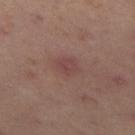Recorded during total-body skin imaging; not selected for excision or biopsy. The tile uses cross-polarized illumination. The lesion-visualizer software estimated an outline eccentricity of about 0.75 (0 = round, 1 = elongated). The software also gave a lesion–skin lightness drop of about 5 and a lesion-to-skin contrast of about 4.5 (normalized; higher = more distinct). The software also gave a border-irregularity rating of about 2/10, internal color variation of about 3 on a 0–10 scale, and radial color variation of about 1. The software also gave a detector confidence of about 100 out of 100 that the crop contains a lesion. The subject is a female aged approximately 40. The lesion is located on the left thigh. A 15 mm close-up extracted from a 3D total-body photography capture.